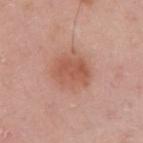biopsy status=total-body-photography surveillance lesion; no biopsy | tile lighting=white-light | size=≈4.5 mm | anatomic site=the right upper arm | patient=female, in their 40s | image=~15 mm crop, total-body skin-cancer survey | image-analysis metrics=a symmetry-axis asymmetry near 0.2; a lesion color around L≈56 a*≈24 b*≈30 in CIELAB, a lesion–skin lightness drop of about 9, and a normalized border contrast of about 6.5.